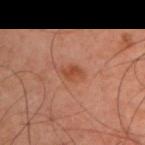biopsy_status: not biopsied; imaged during a skin examination
image:
  source: total-body photography crop
  field_of_view_mm: 15
patient:
  sex: male
  age_approx: 45
lesion_size:
  long_diameter_mm_approx: 2.5
lighting: cross-polarized
site: right upper arm
automated_metrics:
  area_mm2_approx: 3.0
  shape_asymmetry: 0.4
  nevus_likeness_0_100: 85
  lesion_detection_confidence_0_100: 100